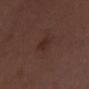No biopsy was performed on this lesion — it was imaged during a full skin examination and was not determined to be concerning. A female subject, aged 48–52. Imaged with white-light lighting. Located on the chest. A lesion tile, about 15 mm wide, cut from a 3D total-body photograph.